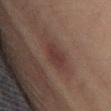biopsy_status: not biopsied; imaged during a skin examination
patient:
  sex: male
  age_approx: 40
automated_metrics:
  area_mm2_approx: 5.0
  eccentricity: 0.8
  shape_asymmetry: 0.3
  nevus_likeness_0_100: 20
site: abdomen
lesion_size:
  long_diameter_mm_approx: 3.5
image:
  source: total-body photography crop
  field_of_view_mm: 15
lighting: cross-polarized